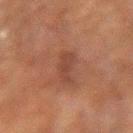<lesion>
  <biopsy_status>not biopsied; imaged during a skin examination</biopsy_status>
  <image>
    <source>total-body photography crop</source>
    <field_of_view_mm>15</field_of_view_mm>
  </image>
  <patient>
    <sex>male</sex>
    <age_approx>60</age_approx>
  </patient>
  <site>left arm</site>
</lesion>